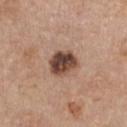follow-up=imaged on a skin check; not biopsied | lesion size=~3.5 mm (longest diameter) | subject=female, approximately 45 years of age | anatomic site=the chest | image=15 mm crop, total-body photography | tile lighting=white-light illumination.